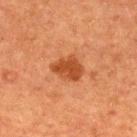This lesion was catalogued during total-body skin photography and was not selected for biopsy.
A 15 mm close-up tile from a total-body photography series done for melanoma screening.
A male subject, aged around 50.
The tile uses cross-polarized illumination.
Measured at roughly 4 mm in maximum diameter.
The lesion is located on the upper back.